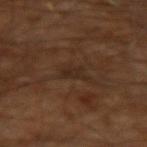Notes:
- biopsy status · total-body-photography surveillance lesion; no biopsy
- tile lighting · cross-polarized
- location · the left arm
- imaging modality · ~15 mm tile from a whole-body skin photo
- patient · male, roughly 60 years of age
- size · ≈2.5 mm